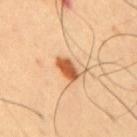Impression:
The lesion was photographed on a routine skin check and not biopsied; there is no pathology result.
Context:
A 15 mm close-up extracted from a 3D total-body photography capture. The lesion is located on the mid back. This is a cross-polarized tile. A male patient aged 63–67. About 3.5 mm across.